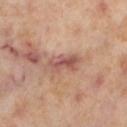| feature | finding |
|---|---|
| notes | total-body-photography surveillance lesion; no biopsy |
| patient | female, about 55 years old |
| location | the left lower leg |
| diameter | ~5 mm (longest diameter) |
| acquisition | ~15 mm crop, total-body skin-cancer survey |
| tile lighting | cross-polarized |